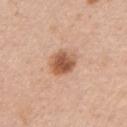workup: no biopsy performed (imaged during a skin exam) | TBP lesion metrics: a lesion area of about 8 mm², an outline eccentricity of about 0.45 (0 = round, 1 = elongated), and two-axis asymmetry of about 0.1; border irregularity of about 1 on a 0–10 scale, a within-lesion color-variation index near 5/10, and peripheral color asymmetry of about 1.5; a classifier nevus-likeness of about 100/100 and lesion-presence confidence of about 100/100 | anatomic site: the left upper arm | subject: female, roughly 45 years of age | illumination: white-light illumination | size: about 3.5 mm | image source: ~15 mm crop, total-body skin-cancer survey.